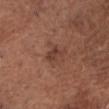Imaged during a routine full-body skin examination; the lesion was not biopsied and no histopathology is available.